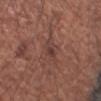Clinical impression:
Part of a total-body skin-imaging series; this lesion was reviewed on a skin check and was not flagged for biopsy.
Clinical summary:
Automated image analysis of the tile measured an average lesion color of about L≈39 a*≈20 b*≈22 (CIELAB), roughly 6 lightness units darker than nearby skin, and a lesion-to-skin contrast of about 6 (normalized; higher = more distinct). It also reported a nevus-likeness score of about 0/100. From the left forearm. This image is a 15 mm lesion crop taken from a total-body photograph. Longest diameter approximately 2.5 mm. The subject is a male aged approximately 55.